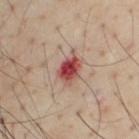The lesion was tiled from a total-body skin photograph and was not biopsied. The subject is a male aged around 55. The lesion-visualizer software estimated an area of roughly 7.5 mm². The software also gave a lesion color around L≈49 a*≈28 b*≈25 in CIELAB, roughly 15 lightness units darker than nearby skin, and a lesion-to-skin contrast of about 10.5 (normalized; higher = more distinct). It also reported a border-irregularity index near 3.5/10 and a peripheral color-asymmetry measure near 2.5. The lesion's longest dimension is about 4 mm. This is a cross-polarized tile. The lesion is located on the chest. This image is a 15 mm lesion crop taken from a total-body photograph.